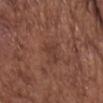size = ~3 mm (longest diameter) | lighting = white-light illumination | automated metrics = a lesion area of about 3 mm² and an eccentricity of roughly 0.9; a lesion color around L≈37 a*≈21 b*≈25 in CIELAB, roughly 6 lightness units darker than nearby skin, and a normalized border contrast of about 5.5; a border-irregularity rating of about 5.5/10, a within-lesion color-variation index near 0/10, and radial color variation of about 0; a classifier nevus-likeness of about 0/100 and a lesion-detection confidence of about 100/100 | image source = total-body-photography crop, ~15 mm field of view | patient = male, roughly 75 years of age | site = the chest.